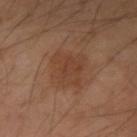Assessment: The lesion was photographed on a routine skin check and not biopsied; there is no pathology result. Image and clinical context: A male patient in their mid- to late 40s. Captured under cross-polarized illumination. From the left forearm. This image is a 15 mm lesion crop taken from a total-body photograph. An algorithmic analysis of the crop reported a lesion area of about 13 mm², a shape eccentricity near 0.7, and two-axis asymmetry of about 0.25. And it measured a border-irregularity index near 3.5/10 and internal color variation of about 3 on a 0–10 scale. And it measured a detector confidence of about 100 out of 100 that the crop contains a lesion. About 5 mm across.